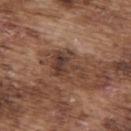Q: Is there a histopathology result?
A: no biopsy performed (imaged during a skin exam)
Q: What are the patient's age and sex?
A: male, about 75 years old
Q: Lesion location?
A: the upper back
Q: What kind of image is this?
A: ~15 mm crop, total-body skin-cancer survey
Q: Lesion size?
A: ~4.5 mm (longest diameter)
Q: Automated lesion metrics?
A: a border-irregularity rating of about 7/10 and a color-variation rating of about 5.5/10; lesion-presence confidence of about 95/100